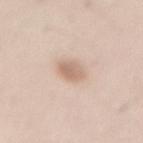Assessment:
Imaged during a routine full-body skin examination; the lesion was not biopsied and no histopathology is available.
Image and clinical context:
Approximately 2.5 mm at its widest. Located on the lower back. A male patient, approximately 55 years of age. This is a white-light tile. A region of skin cropped from a whole-body photographic capture, roughly 15 mm wide.